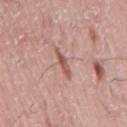No biopsy was performed on this lesion — it was imaged during a full skin examination and was not determined to be concerning. The lesion is on the mid back. The total-body-photography lesion software estimated border irregularity of about 3 on a 0–10 scale, internal color variation of about 0 on a 0–10 scale, and a peripheral color-asymmetry measure near 0. The software also gave a classifier nevus-likeness of about 5/100 and lesion-presence confidence of about 90/100. Imaged with white-light lighting. A male subject, aged around 75. The recorded lesion diameter is about 3.5 mm. Cropped from a whole-body photographic skin survey; the tile spans about 15 mm.